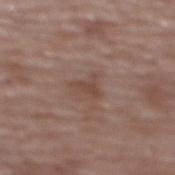follow-up — no biopsy performed (imaged during a skin exam)
lighting — white-light illumination
image — total-body-photography crop, ~15 mm field of view
patient — female, about 65 years old
anatomic site — the upper back
automated lesion analysis — a lesion–skin lightness drop of about 7 and a lesion-to-skin contrast of about 5.5 (normalized; higher = more distinct)